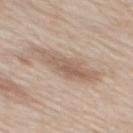{"biopsy_status": "not biopsied; imaged during a skin examination", "lighting": "white-light", "site": "mid back", "lesion_size": {"long_diameter_mm_approx": 7.0}, "image": {"source": "total-body photography crop", "field_of_view_mm": 15}, "patient": {"sex": "male", "age_approx": 60}}